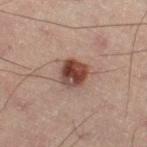Case summary:
- patient — male, roughly 50 years of age
- site — the right thigh
- imaging modality — total-body-photography crop, ~15 mm field of view
- illumination — cross-polarized
- size — ≈3.5 mm